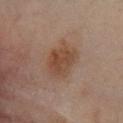biopsy status = catalogued during a skin exam; not biopsied
imaging modality = 15 mm crop, total-body photography
patient = male, about 65 years old
lighting = cross-polarized illumination
automated metrics = an area of roughly 13 mm² and a symmetry-axis asymmetry near 0.15; a lesion color around L≈43 a*≈17 b*≈27 in CIELAB, a lesion–skin lightness drop of about 8, and a normalized lesion–skin contrast near 7.5; a color-variation rating of about 3/10 and a peripheral color-asymmetry measure near 1
location = the right lower leg